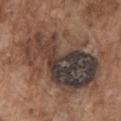The lesion was tiled from a total-body skin photograph and was not biopsied.
The patient is a male aged approximately 75.
Automated tile analysis of the lesion measured a lesion color around L≈40 a*≈14 b*≈21 in CIELAB, roughly 12 lightness units darker than nearby skin, and a normalized border contrast of about 11. The analysis additionally found a nevus-likeness score of about 0/100.
The lesion is located on the chest.
Captured under white-light illumination.
Cropped from a whole-body photographic skin survey; the tile spans about 15 mm.
The lesion's longest dimension is about 11.5 mm.